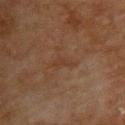Case summary:
* follow-up · total-body-photography surveillance lesion; no biopsy
* acquisition · total-body-photography crop, ~15 mm field of view
* body site · the upper back
* lighting · cross-polarized
* subject · female, aged around 80